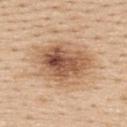The lesion was photographed on a routine skin check and not biopsied; there is no pathology result. The patient is a female aged approximately 45. A roughly 15 mm field-of-view crop from a total-body skin photograph. On the upper back.